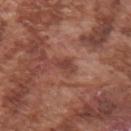Notes:
– workup · no biopsy performed (imaged during a skin exam)
– subject · male, in their mid- to late 70s
– acquisition · ~15 mm tile from a whole-body skin photo
– anatomic site · the arm